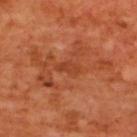| feature | finding |
|---|---|
| biopsy status | total-body-photography surveillance lesion; no biopsy |
| subject | male, approximately 65 years of age |
| diameter | about 2.5 mm |
| anatomic site | the upper back |
| acquisition | total-body-photography crop, ~15 mm field of view |
| tile lighting | cross-polarized illumination |